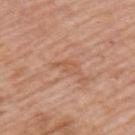- body site · the upper back
- image source · ~15 mm tile from a whole-body skin photo
- diameter · ~3.5 mm (longest diameter)
- subject · male, approximately 75 years of age
- illumination · white-light